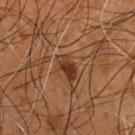Part of a total-body skin-imaging series; this lesion was reviewed on a skin check and was not flagged for biopsy. Cropped from a whole-body photographic skin survey; the tile spans about 15 mm. A male subject, in their mid- to late 50s. Automated image analysis of the tile measured a mean CIELAB color near L≈33 a*≈21 b*≈29, roughly 10 lightness units darker than nearby skin, and a normalized lesion–skin contrast near 9. The lesion's longest dimension is about 3.5 mm. The lesion is on the chest.